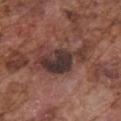| feature | finding |
|---|---|
| workup | imaged on a skin check; not biopsied |
| subject | male, aged 73–77 |
| acquisition | ~15 mm crop, total-body skin-cancer survey |
| site | the chest |